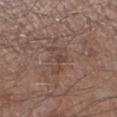Assessment: The lesion was tiled from a total-body skin photograph and was not biopsied. Image and clinical context: Automated image analysis of the tile measured a lesion area of about 5 mm² and a shape eccentricity near 0.85. It also reported a mean CIELAB color near L≈43 a*≈17 b*≈23. It also reported an automated nevus-likeness rating near 0 out of 100 and lesion-presence confidence of about 95/100. Cropped from a whole-body photographic skin survey; the tile spans about 15 mm. This is a white-light tile. A male subject, in their mid- to late 50s. The lesion is located on the left lower leg.